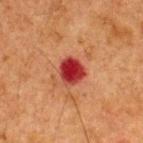follow-up: imaged on a skin check; not biopsied
body site: the back
automated lesion analysis: an average lesion color of about L≈35 a*≈35 b*≈29 (CIELAB) and about 14 CIELAB-L* units darker than the surrounding skin; a border-irregularity rating of about 2/10, a within-lesion color-variation index near 5/10, and radial color variation of about 1.5
acquisition: ~15 mm tile from a whole-body skin photo
lighting: cross-polarized illumination
patient: male, roughly 65 years of age